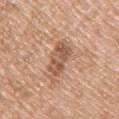Recorded during total-body skin imaging; not selected for excision or biopsy. From the left arm. About 5.5 mm across. A male patient aged 78–82. The lesion-visualizer software estimated a footprint of about 10 mm², an eccentricity of roughly 0.85, and a symmetry-axis asymmetry near 0.25. The analysis additionally found an average lesion color of about L≈56 a*≈21 b*≈30 (CIELAB), roughly 11 lightness units darker than nearby skin, and a lesion-to-skin contrast of about 7 (normalized; higher = more distinct). The analysis additionally found an automated nevus-likeness rating near 0 out of 100. A 15 mm close-up extracted from a 3D total-body photography capture.